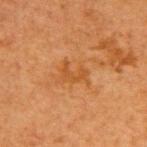Notes:
– follow-up · no biopsy performed (imaged during a skin exam)
– body site · the upper back
– diameter · ≈3 mm
– acquisition · ~15 mm tile from a whole-body skin photo
– automated lesion analysis · a footprint of about 3.5 mm² and an eccentricity of roughly 0.8; a border-irregularity rating of about 7.5/10 and a within-lesion color-variation index near 0/10
– lighting · cross-polarized
– subject · female, aged 38 to 42